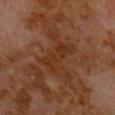The recorded lesion diameter is about 5.5 mm.
An algorithmic analysis of the crop reported border irregularity of about 5.5 on a 0–10 scale and a color-variation rating of about 2/10. The software also gave an automated nevus-likeness rating near 0 out of 100 and a lesion-detection confidence of about 95/100.
Cropped from a total-body skin-imaging series; the visible field is about 15 mm.
Located on the chest.
The patient is a male approximately 80 years of age.